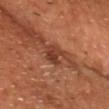Notes:
– workup — total-body-photography surveillance lesion; no biopsy
– patient — male, aged 63–67
– tile lighting — cross-polarized
– image — total-body-photography crop, ~15 mm field of view
– image-analysis metrics — a shape eccentricity near 0.3 and a shape-asymmetry score of about 0.3 (0 = symmetric); a lesion color around L≈38 a*≈25 b*≈31 in CIELAB, roughly 10 lightness units darker than nearby skin, and a lesion-to-skin contrast of about 8 (normalized; higher = more distinct); an automated nevus-likeness rating near 40 out of 100 and a lesion-detection confidence of about 100/100
– diameter — about 2.5 mm